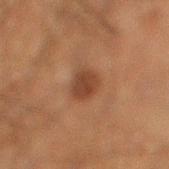Notes:
- follow-up — no biopsy performed (imaged during a skin exam)